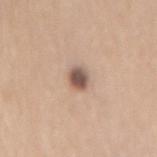| key | value |
|---|---|
| notes | catalogued during a skin exam; not biopsied |
| diameter | about 2.5 mm |
| subject | female, about 70 years old |
| tile lighting | white-light illumination |
| body site | the mid back |
| image source | total-body-photography crop, ~15 mm field of view |